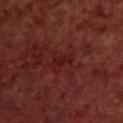The lesion was photographed on a routine skin check and not biopsied; there is no pathology result. The patient is a male aged approximately 70. The lesion is located on the upper back. Captured under cross-polarized illumination. A roughly 15 mm field-of-view crop from a total-body skin photograph. Approximately 3 mm at its widest.